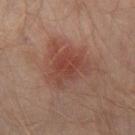The lesion was tiled from a total-body skin photograph and was not biopsied. A region of skin cropped from a whole-body photographic capture, roughly 15 mm wide. On the left leg. A male subject, aged around 30. The total-body-photography lesion software estimated an average lesion color of about L≈41 a*≈24 b*≈25 (CIELAB) and a normalized lesion–skin contrast near 6. This is a cross-polarized tile.